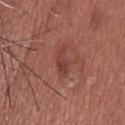Recorded during total-body skin imaging; not selected for excision or biopsy. An algorithmic analysis of the crop reported a lesion area of about 4 mm² and an outline eccentricity of about 0.9 (0 = round, 1 = elongated). The software also gave a border-irregularity index near 4/10 and internal color variation of about 1.5 on a 0–10 scale. The software also gave a classifier nevus-likeness of about 0/100 and a lesion-detection confidence of about 100/100. A male patient, aged around 50. The lesion is on the upper back. Cropped from a whole-body photographic skin survey; the tile spans about 15 mm.